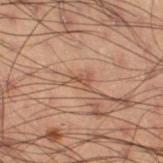The lesion was tiled from a total-body skin photograph and was not biopsied. A 15 mm close-up extracted from a 3D total-body photography capture. The subject is a male aged 53–57. Located on the right thigh.